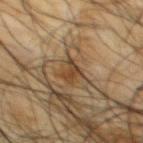- follow-up · catalogued during a skin exam; not biopsied
- anatomic site · the upper back
- acquisition · 15 mm crop, total-body photography
- illumination · cross-polarized illumination
- patient · male, roughly 65 years of age
- automated lesion analysis · a lesion area of about 3.5 mm², an eccentricity of roughly 0.85, and two-axis asymmetry of about 0.4; an average lesion color of about L≈40 a*≈17 b*≈32 (CIELAB), roughly 9 lightness units darker than nearby skin, and a normalized lesion–skin contrast near 8; a border-irregularity rating of about 4.5/10, a color-variation rating of about 2.5/10, and a peripheral color-asymmetry measure near 0.5